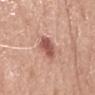workup: total-body-photography surveillance lesion; no biopsy | image source: total-body-photography crop, ~15 mm field of view | lighting: white-light | body site: the mid back | lesion size: about 4 mm | subject: male, about 50 years old.